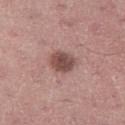workup: no biopsy performed (imaged during a skin exam)
patient: male, aged 58 to 62
size: about 3.5 mm
image-analysis metrics: a lesion color around L≈49 a*≈21 b*≈22 in CIELAB, roughly 13 lightness units darker than nearby skin, and a normalized border contrast of about 9
anatomic site: the right thigh
illumination: white-light illumination
image: total-body-photography crop, ~15 mm field of view